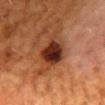Part of a total-body skin-imaging series; this lesion was reviewed on a skin check and was not flagged for biopsy. Approximately 3.5 mm at its widest. This image is a 15 mm lesion crop taken from a total-body photograph. On the mid back. The lesion-visualizer software estimated a footprint of about 9 mm², an eccentricity of roughly 0.5, and a shape-asymmetry score of about 0.2 (0 = symmetric). The software also gave a lesion color around L≈24 a*≈21 b*≈26 in CIELAB, a lesion–skin lightness drop of about 15, and a normalized lesion–skin contrast near 14.5. A female subject aged 48–52. Captured under cross-polarized illumination.